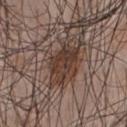Imaged with white-light lighting. A roughly 15 mm field-of-view crop from a total-body skin photograph. The recorded lesion diameter is about 4.5 mm. A male subject, in their mid-40s. The lesion is located on the chest.